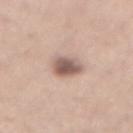| feature | finding |
|---|---|
| biopsy status | imaged on a skin check; not biopsied |
| image source | ~15 mm tile from a whole-body skin photo |
| patient | male, aged approximately 35 |
| body site | the back |
| lighting | white-light illumination |
| TBP lesion metrics | an area of roughly 6.5 mm², a shape eccentricity near 0.5, and a symmetry-axis asymmetry near 0.2; an average lesion color of about L≈57 a*≈18 b*≈23 (CIELAB), a lesion–skin lightness drop of about 15, and a normalized border contrast of about 9.5 |